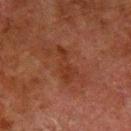| key | value |
|---|---|
| follow-up | no biopsy performed (imaged during a skin exam) |
| lesion diameter | ~4.5 mm (longest diameter) |
| patient | male, aged approximately 80 |
| TBP lesion metrics | an area of roughly 5.5 mm²; an average lesion color of about L≈27 a*≈21 b*≈26 (CIELAB), about 5 CIELAB-L* units darker than the surrounding skin, and a normalized lesion–skin contrast near 6; a border-irregularity rating of about 5/10, a within-lesion color-variation index near 1.5/10, and radial color variation of about 0.5 |
| body site | the left lower leg |
| lighting | cross-polarized illumination |
| image | ~15 mm crop, total-body skin-cancer survey |